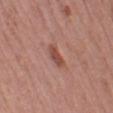Impression:
No biopsy was performed on this lesion — it was imaged during a full skin examination and was not determined to be concerning.
Context:
Captured under white-light illumination. A close-up tile cropped from a whole-body skin photograph, about 15 mm across. The lesion's longest dimension is about 3 mm. The lesion is located on the left thigh. A female patient, aged around 40.